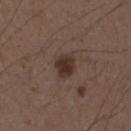Impression: The lesion was tiled from a total-body skin photograph and was not biopsied. Context: Automated image analysis of the tile measured a footprint of about 6 mm², an eccentricity of roughly 0.55, and a symmetry-axis asymmetry near 0.15. The analysis additionally found a mean CIELAB color near L≈32 a*≈15 b*≈21 and a lesion-to-skin contrast of about 9.5 (normalized; higher = more distinct). It also reported a border-irregularity rating of about 1.5/10. A lesion tile, about 15 mm wide, cut from a 3D total-body photograph. The lesion is on the arm. Measured at roughly 3 mm in maximum diameter. The subject is a male approximately 50 years of age.